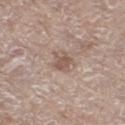Q: Lesion location?
A: the right thigh
Q: What are the patient's age and sex?
A: female, aged 73 to 77
Q: How was the tile lit?
A: white-light illumination
Q: Lesion size?
A: ~3 mm (longest diameter)
Q: How was this image acquired?
A: ~15 mm tile from a whole-body skin photo